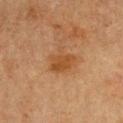biopsy status — total-body-photography surveillance lesion; no biopsy | patient — female, aged approximately 50 | anatomic site — the chest | TBP lesion metrics — an area of roughly 7 mm², an eccentricity of roughly 0.55, and a shape-asymmetry score of about 0.35 (0 = symmetric); an average lesion color of about L≈41 a*≈20 b*≈35 (CIELAB) and a normalized border contrast of about 7; an automated nevus-likeness rating near 70 out of 100 and a detector confidence of about 100 out of 100 that the crop contains a lesion | acquisition — ~15 mm crop, total-body skin-cancer survey.